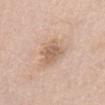Impression: The lesion was tiled from a total-body skin photograph and was not biopsied. Image and clinical context: The lesion-visualizer software estimated a lesion area of about 7.5 mm². And it measured a border-irregularity rating of about 2.5/10, a color-variation rating of about 2.5/10, and radial color variation of about 1. The software also gave a detector confidence of about 100 out of 100 that the crop contains a lesion. On the front of the torso. The lesion's longest dimension is about 3 mm. A region of skin cropped from a whole-body photographic capture, roughly 15 mm wide. A female subject, approximately 70 years of age.